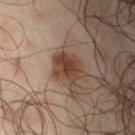Recorded during total-body skin imaging; not selected for excision or biopsy. The tile uses cross-polarized illumination. A male subject, aged 63 to 67. The lesion is on the right thigh. A region of skin cropped from a whole-body photographic capture, roughly 15 mm wide.